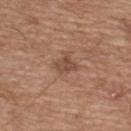  biopsy_status: not biopsied; imaged during a skin examination
  lighting: white-light
  image:
    source: total-body photography crop
    field_of_view_mm: 15
  lesion_size:
    long_diameter_mm_approx: 3.0
  site: upper back
  patient:
    sex: male
    age_approx: 65
  automated_metrics:
    area_mm2_approx: 4.5
    eccentricity: 0.75
    shape_asymmetry: 0.25